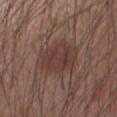Findings:
• follow-up · imaged on a skin check; not biopsied
• illumination · white-light illumination
• acquisition · total-body-photography crop, ~15 mm field of view
• subject · male, in their mid- to late 40s
• diameter · ≈6 mm
• location · the left forearm